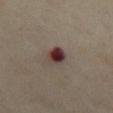follow-up: total-body-photography surveillance lesion; no biopsy | imaging modality: 15 mm crop, total-body photography | body site: the chest | subject: female, aged 53–57.